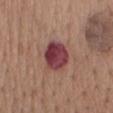Clinical impression:
Recorded during total-body skin imaging; not selected for excision or biopsy.
Acquisition and patient details:
A roughly 15 mm field-of-view crop from a total-body skin photograph. Located on the mid back. The subject is a female aged around 65.